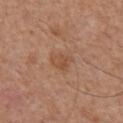Findings:
– notes: total-body-photography surveillance lesion; no biopsy
– patient: male, about 65 years old
– location: the front of the torso
– imaging modality: ~15 mm crop, total-body skin-cancer survey